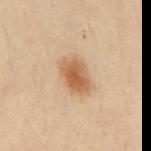{"biopsy_status": "not biopsied; imaged during a skin examination", "site": "abdomen", "patient": {"sex": "male", "age_approx": 30}, "image": {"source": "total-body photography crop", "field_of_view_mm": 15}, "automated_metrics": {"cielab_L": 48, "cielab_a": 16, "cielab_b": 30, "vs_skin_darker_L": 10.0, "vs_skin_contrast_norm": 8.0, "border_irregularity_0_10": 1.5, "peripheral_color_asymmetry": 1.0, "nevus_likeness_0_100": 100, "lesion_detection_confidence_0_100": 100}, "lighting": "cross-polarized"}